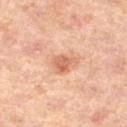Q: Is there a histopathology result?
A: imaged on a skin check; not biopsied
Q: What kind of image is this?
A: ~15 mm crop, total-body skin-cancer survey
Q: Who is the patient?
A: female, about 40 years old
Q: What is the anatomic site?
A: the left lower leg